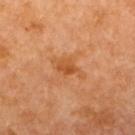This lesion was catalogued during total-body skin photography and was not selected for biopsy. Imaged with cross-polarized lighting. Measured at roughly 4 mm in maximum diameter. The lesion is located on the upper back. Cropped from a whole-body photographic skin survey; the tile spans about 15 mm. A female patient, roughly 50 years of age.